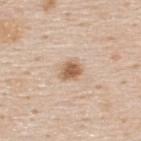biopsy status: no biopsy performed (imaged during a skin exam) | subject: male, about 45 years old | location: the upper back | automated metrics: roughly 13 lightness units darker than nearby skin and a normalized lesion–skin contrast near 9.5; a lesion-detection confidence of about 100/100 | image source: ~15 mm crop, total-body skin-cancer survey | lighting: white-light.